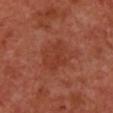Part of a total-body skin-imaging series; this lesion was reviewed on a skin check and was not flagged for biopsy.